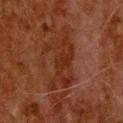notes — catalogued during a skin exam; not biopsied | image source — total-body-photography crop, ~15 mm field of view | subject — male, aged 78–82 | automated lesion analysis — a mean CIELAB color near L≈23 a*≈21 b*≈25, about 5 CIELAB-L* units darker than the surrounding skin, and a normalized border contrast of about 6.5; an automated nevus-likeness rating near 0 out of 100 and lesion-presence confidence of about 85/100 | lesion diameter — ≈7.5 mm | lighting — cross-polarized illumination | body site — the head or neck.